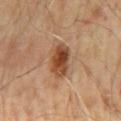<case>
  <biopsy_status>not biopsied; imaged during a skin examination</biopsy_status>
  <patient>
    <sex>male</sex>
    <age_approx>65</age_approx>
  </patient>
  <lighting>cross-polarized</lighting>
  <image>
    <source>total-body photography crop</source>
    <field_of_view_mm>15</field_of_view_mm>
  </image>
  <site>mid back</site>
  <lesion_size>
    <long_diameter_mm_approx>4.5</long_diameter_mm_approx>
  </lesion_size>
</case>